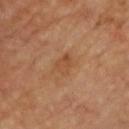Case summary:
* biopsy status · catalogued during a skin exam; not biopsied
* patient · male, approximately 60 years of age
* location · the chest
* image · ~15 mm tile from a whole-body skin photo
* tile lighting · cross-polarized
* diameter · ~2.5 mm (longest diameter)